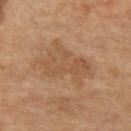Part of a total-body skin-imaging series; this lesion was reviewed on a skin check and was not flagged for biopsy. Located on the left thigh. A 15 mm crop from a total-body photograph taken for skin-cancer surveillance. The patient is a female approximately 55 years of age.